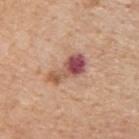Q: Was this lesion biopsied?
A: no biopsy performed (imaged during a skin exam)
Q: What is the imaging modality?
A: ~15 mm crop, total-body skin-cancer survey
Q: Where on the body is the lesion?
A: the upper back
Q: What is the lesion's diameter?
A: about 5 mm
Q: Illumination type?
A: white-light
Q: What did automated image analysis measure?
A: a shape eccentricity near 0.9 and two-axis asymmetry of about 0.4; border irregularity of about 4.5 on a 0–10 scale, internal color variation of about 9.5 on a 0–10 scale, and peripheral color asymmetry of about 3; lesion-presence confidence of about 100/100
Q: Who is the patient?
A: male, about 60 years old